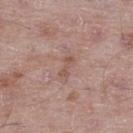{"biopsy_status": "not biopsied; imaged during a skin examination", "automated_metrics": {"area_mm2_approx": 2.5, "eccentricity": 0.9, "shape_asymmetry": 0.4, "border_irregularity_0_10": 5.0, "color_variation_0_10": 0.0, "peripheral_color_asymmetry": 0.0, "nevus_likeness_0_100": 0, "lesion_detection_confidence_0_100": 100}, "lighting": "white-light", "image": {"source": "total-body photography crop", "field_of_view_mm": 15}, "site": "right thigh", "patient": {"sex": "male", "age_approx": 50}, "lesion_size": {"long_diameter_mm_approx": 2.5}}